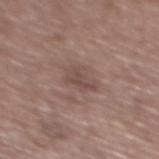Recorded during total-body skin imaging; not selected for excision or biopsy.